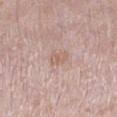notes — imaged on a skin check; not biopsied | lesion diameter — ~2.5 mm (longest diameter) | site — the leg | image — ~15 mm tile from a whole-body skin photo | patient — female, roughly 40 years of age | illumination — white-light.